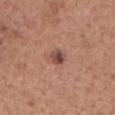biopsy_status: not biopsied; imaged during a skin examination
lesion_size:
  long_diameter_mm_approx: 2.5
patient:
  sex: male
  age_approx: 65
lighting: white-light
site: head or neck
image:
  source: total-body photography crop
  field_of_view_mm: 15
automated_metrics:
  vs_skin_darker_L: 12.0
  vs_skin_contrast_norm: 9.0
  border_irregularity_0_10: 2.0
  color_variation_0_10: 6.5
  peripheral_color_asymmetry: 2.5